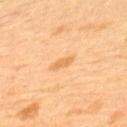This lesion was catalogued during total-body skin photography and was not selected for biopsy. The recorded lesion diameter is about 3 mm. This is a cross-polarized tile. A 15 mm crop from a total-body photograph taken for skin-cancer surveillance. From the upper back. Automated tile analysis of the lesion measured a mean CIELAB color near L≈67 a*≈23 b*≈44 and a normalized lesion–skin contrast near 6. The analysis additionally found border irregularity of about 3 on a 0–10 scale, a within-lesion color-variation index near 0/10, and peripheral color asymmetry of about 0. It also reported a classifier nevus-likeness of about 10/100 and lesion-presence confidence of about 100/100. The subject is a male aged 63–67.